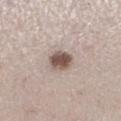Impression:
The lesion was photographed on a routine skin check and not biopsied; there is no pathology result.
Acquisition and patient details:
Captured under white-light illumination. On the leg. The lesion's longest dimension is about 3 mm. A female subject approximately 40 years of age. An algorithmic analysis of the crop reported a footprint of about 6.5 mm² and a symmetry-axis asymmetry near 0.15. The software also gave an average lesion color of about L≈52 a*≈15 b*≈21 (CIELAB), about 16 CIELAB-L* units darker than the surrounding skin, and a normalized lesion–skin contrast near 11. It also reported a nevus-likeness score of about 95/100 and a detector confidence of about 100 out of 100 that the crop contains a lesion. A 15 mm crop from a total-body photograph taken for skin-cancer surveillance.